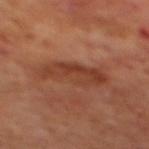follow-up: catalogued during a skin exam; not biopsied | lighting: cross-polarized | body site: the mid back | patient: male, in their 70s | image source: 15 mm crop, total-body photography.